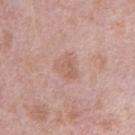| field | value |
|---|---|
| notes | no biopsy performed (imaged during a skin exam) |
| patient | male, in their 40s |
| automated metrics | an average lesion color of about L≈60 a*≈21 b*≈27 (CIELAB), a lesion–skin lightness drop of about 7, and a normalized border contrast of about 5.5; a within-lesion color-variation index near 2/10 and radial color variation of about 1; a detector confidence of about 100 out of 100 that the crop contains a lesion |
| image | 15 mm crop, total-body photography |
| body site | the right upper arm |
| illumination | white-light |